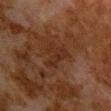| field | value |
|---|---|
| biopsy status | no biopsy performed (imaged during a skin exam) |
| illumination | cross-polarized illumination |
| image | total-body-photography crop, ~15 mm field of view |
| image-analysis metrics | an automated nevus-likeness rating near 0 out of 100 and a lesion-detection confidence of about 90/100 |
| anatomic site | the chest |
| subject | male, aged approximately 80 |